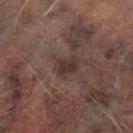Imaged during a routine full-body skin examination; the lesion was not biopsied and no histopathology is available. Automated image analysis of the tile measured a footprint of about 5 mm², an outline eccentricity of about 0.65 (0 = round, 1 = elongated), and a shape-asymmetry score of about 0.3 (0 = symmetric). The analysis additionally found a lesion color around L≈25 a*≈12 b*≈15 in CIELAB. And it measured a border-irregularity rating of about 3/10, a within-lesion color-variation index near 2.5/10, and a peripheral color-asymmetry measure near 1. Imaged with cross-polarized lighting. This image is a 15 mm lesion crop taken from a total-body photograph. On the leg. A male patient aged approximately 75. Longest diameter approximately 3 mm.